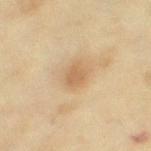Clinical impression:
No biopsy was performed on this lesion — it was imaged during a full skin examination and was not determined to be concerning.
Background:
The lesion-visualizer software estimated border irregularity of about 2 on a 0–10 scale and a within-lesion color-variation index near 1/10. On the left thigh. A lesion tile, about 15 mm wide, cut from a 3D total-body photograph. The tile uses cross-polarized illumination. The subject is a female in their mid- to late 50s.